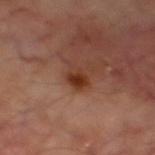Notes:
• follow-up: total-body-photography surveillance lesion; no biopsy
• image source: ~15 mm crop, total-body skin-cancer survey
• lesion diameter: ~3 mm (longest diameter)
• automated metrics: a lesion-to-skin contrast of about 9.5 (normalized; higher = more distinct); a nevus-likeness score of about 95/100 and a detector confidence of about 100 out of 100 that the crop contains a lesion
• tile lighting: cross-polarized illumination
• patient: male, aged approximately 70
• site: the leg